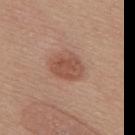Q: Was this lesion biopsied?
A: total-body-photography surveillance lesion; no biopsy
Q: What is the anatomic site?
A: the back
Q: Automated lesion metrics?
A: a lesion area of about 9 mm², an outline eccentricity of about 0.65 (0 = round, 1 = elongated), and a symmetry-axis asymmetry near 0.2; a nevus-likeness score of about 90/100 and a detector confidence of about 100 out of 100 that the crop contains a lesion
Q: What is the imaging modality?
A: ~15 mm tile from a whole-body skin photo
Q: Patient demographics?
A: female, about 65 years old
Q: What lighting was used for the tile?
A: white-light